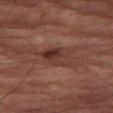No biopsy was performed on this lesion — it was imaged during a full skin examination and was not determined to be concerning.
Captured under cross-polarized illumination.
Measured at roughly 4.5 mm in maximum diameter.
On the right thigh.
A lesion tile, about 15 mm wide, cut from a 3D total-body photograph.
A male subject about 85 years old or older.